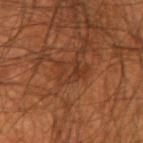The lesion was tiled from a total-body skin photograph and was not biopsied.
The lesion is located on the arm.
The tile uses cross-polarized illumination.
A roughly 15 mm field-of-view crop from a total-body skin photograph.
A male subject in their mid-40s.
Measured at roughly 4 mm in maximum diameter.